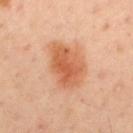| field | value |
|---|---|
| biopsy status | total-body-photography surveillance lesion; no biopsy |
| TBP lesion metrics | an area of roughly 16 mm², an outline eccentricity of about 0.7 (0 = round, 1 = elongated), and two-axis asymmetry of about 0.2 |
| subject | male, aged approximately 45 |
| anatomic site | the mid back |
| diameter | ≈5.5 mm |
| acquisition | total-body-photography crop, ~15 mm field of view |